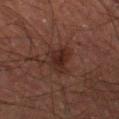biopsy_status: not biopsied; imaged during a skin examination
automated_metrics:
  area_mm2_approx: 8.0
  eccentricity: 0.45
  shape_asymmetry: 0.35
  vs_skin_darker_L: 6.0
site: left thigh
patient:
  sex: male
  age_approx: 50
image:
  source: total-body photography crop
  field_of_view_mm: 15
lighting: cross-polarized
lesion_size:
  long_diameter_mm_approx: 3.5